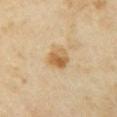Notes:
• workup: imaged on a skin check; not biopsied
• automated metrics: a mean CIELAB color near L≈59 a*≈16 b*≈38, about 10 CIELAB-L* units darker than the surrounding skin, and a lesion-to-skin contrast of about 8 (normalized; higher = more distinct); border irregularity of about 2 on a 0–10 scale and internal color variation of about 6.5 on a 0–10 scale
• location: the left upper arm
• illumination: cross-polarized
• imaging modality: 15 mm crop, total-body photography
• subject: female, about 35 years old